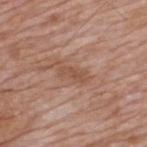{
  "image": {
    "source": "total-body photography crop",
    "field_of_view_mm": 15
  },
  "site": "upper back",
  "patient": {
    "sex": "male",
    "age_approx": 60
  }
}